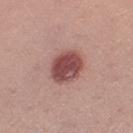Impression:
This lesion was catalogued during total-body skin photography and was not selected for biopsy.
Acquisition and patient details:
Cropped from a whole-body photographic skin survey; the tile spans about 15 mm. Located on the right thigh. A female subject aged 23–27. The tile uses white-light illumination. Approximately 4.5 mm at its widest.